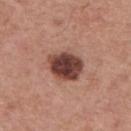Clinical impression: Recorded during total-body skin imaging; not selected for excision or biopsy. Acquisition and patient details: The total-body-photography lesion software estimated a lesion area of about 13 mm² and two-axis asymmetry of about 0.15. A male subject, aged approximately 65. The lesion is on the upper back. The lesion's longest dimension is about 4.5 mm. A close-up tile cropped from a whole-body skin photograph, about 15 mm across.